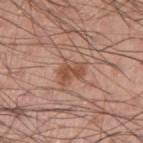Q: Was a biopsy performed?
A: total-body-photography surveillance lesion; no biopsy
Q: What lighting was used for the tile?
A: white-light illumination
Q: Patient demographics?
A: male, approximately 45 years of age
Q: What is the imaging modality?
A: ~15 mm tile from a whole-body skin photo
Q: Where on the body is the lesion?
A: the arm